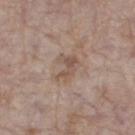The lesion was tiled from a total-body skin photograph and was not biopsied.